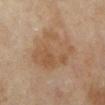Assessment:
Captured during whole-body skin photography for melanoma surveillance; the lesion was not biopsied.
Clinical summary:
A 15 mm close-up extracted from a 3D total-body photography capture. Imaged with cross-polarized lighting. Approximately 7 mm at its widest. The lesion is located on the leg. A female patient, about 70 years old.